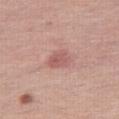biopsy status: no biopsy performed (imaged during a skin exam)
imaging modality: ~15 mm tile from a whole-body skin photo
subject: female, about 50 years old
location: the right thigh